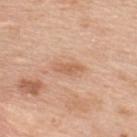The lesion was tiled from a total-body skin photograph and was not biopsied. From the upper back. The tile uses white-light illumination. An algorithmic analysis of the crop reported border irregularity of about 3 on a 0–10 scale, internal color variation of about 1 on a 0–10 scale, and peripheral color asymmetry of about 0.5. It also reported an automated nevus-likeness rating near 0 out of 100 and a detector confidence of about 100 out of 100 that the crop contains a lesion. A 15 mm close-up tile from a total-body photography series done for melanoma screening. A male subject roughly 50 years of age.